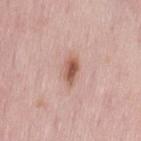<case>
<biopsy_status>not biopsied; imaged during a skin examination</biopsy_status>
<automated_metrics>
  <border_irregularity_0_10>2.0</border_irregularity_0_10>
  <color_variation_0_10>5.5</color_variation_0_10>
  <peripheral_color_asymmetry>2.0</peripheral_color_asymmetry>
</automated_metrics>
<image>
  <source>total-body photography crop</source>
  <field_of_view_mm>15</field_of_view_mm>
</image>
<site>back</site>
<patient>
  <sex>female</sex>
  <age_approx>50</age_approx>
</patient>
<lighting>white-light</lighting>
<lesion_size>
  <long_diameter_mm_approx>3.5</long_diameter_mm_approx>
</lesion_size>
</case>